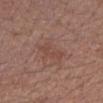<tbp_lesion>
<biopsy_status>not biopsied; imaged during a skin examination</biopsy_status>
<image>
  <source>total-body photography crop</source>
  <field_of_view_mm>15</field_of_view_mm>
</image>
<patient>
  <sex>female</sex>
  <age_approx>55</age_approx>
</patient>
<site>right forearm</site>
</tbp_lesion>